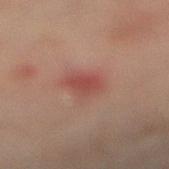{
  "biopsy_status": "not biopsied; imaged during a skin examination"
}